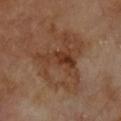Imaged during a routine full-body skin examination; the lesion was not biopsied and no histopathology is available. The tile uses cross-polarized illumination. Cropped from a whole-body photographic skin survey; the tile spans about 15 mm. The lesion's longest dimension is about 9 mm. The lesion is located on the chest. The subject is a male in their 70s.